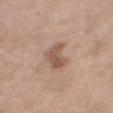biopsy_status: not biopsied; imaged during a skin examination
image:
  source: total-body photography crop
  field_of_view_mm: 15
lesion_size:
  long_diameter_mm_approx: 3.5
lighting: white-light
automated_metrics:
  eccentricity: 0.7
  shape_asymmetry: 0.35
  vs_skin_darker_L: 10.0
  vs_skin_contrast_norm: 7.0
patient:
  sex: female
  age_approx: 55
site: right thigh